Automated image analysis of the tile measured a color-variation rating of about 4.5/10 and peripheral color asymmetry of about 1.5. The software also gave a nevus-likeness score of about 90/100 and lesion-presence confidence of about 100/100; a female subject aged approximately 65; this is a white-light tile; a lesion tile, about 15 mm wide, cut from a 3D total-body photograph; on the left lower leg; longest diameter approximately 7 mm — 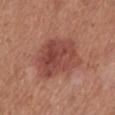On excision, pathology confirmed a skin cancer: superficial basal cell carcinoma.